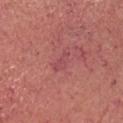{
  "biopsy_status": "not biopsied; imaged during a skin examination",
  "patient": {
    "sex": "male",
    "age_approx": 65
  },
  "lesion_size": {
    "long_diameter_mm_approx": 3.0
  },
  "automated_metrics": {
    "area_mm2_approx": 3.5,
    "eccentricity": 0.9,
    "vs_skin_contrast_norm": 5.0
  },
  "image": {
    "source": "total-body photography crop",
    "field_of_view_mm": 15
  },
  "site": "head or neck",
  "lighting": "white-light"
}